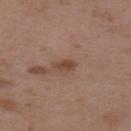follow-up: total-body-photography surveillance lesion; no biopsy | subject: female, aged approximately 35 | diameter: ~2.5 mm (longest diameter) | image: 15 mm crop, total-body photography | tile lighting: white-light | site: the upper back.